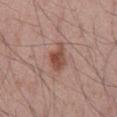{
  "biopsy_status": "not biopsied; imaged during a skin examination",
  "lighting": "white-light",
  "image": {
    "source": "total-body photography crop",
    "field_of_view_mm": 15
  },
  "patient": {
    "sex": "male",
    "age_approx": 45
  },
  "site": "mid back"
}